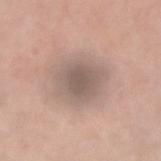Impression:
No biopsy was performed on this lesion — it was imaged during a full skin examination and was not determined to be concerning.
Image and clinical context:
Automated tile analysis of the lesion measured a lesion color around L≈57 a*≈14 b*≈22 in CIELAB, roughly 10 lightness units darker than nearby skin, and a lesion-to-skin contrast of about 7 (normalized; higher = more distinct). Imaged with white-light lighting. A region of skin cropped from a whole-body photographic capture, roughly 15 mm wide. The lesion is on the right upper arm. The patient is a male approximately 55 years of age.